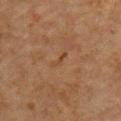biopsy status = no biopsy performed (imaged during a skin exam); subject = male, approximately 60 years of age; lighting = cross-polarized; location = the chest; image = ~15 mm tile from a whole-body skin photo.